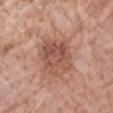The lesion was photographed on a routine skin check and not biopsied; there is no pathology result. The total-body-photography lesion software estimated a footprint of about 15 mm² and a shape-asymmetry score of about 0.25 (0 = symmetric). The software also gave border irregularity of about 3.5 on a 0–10 scale, internal color variation of about 5 on a 0–10 scale, and a peripheral color-asymmetry measure near 2. And it measured an automated nevus-likeness rating near 30 out of 100 and a detector confidence of about 100 out of 100 that the crop contains a lesion. Captured under white-light illumination. About 5 mm across. A close-up tile cropped from a whole-body skin photograph, about 15 mm across. The lesion is on the arm. The patient is a female aged approximately 70.